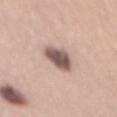notes: no biopsy performed (imaged during a skin exam)
lesion diameter: ≈5 mm
location: the back
imaging modality: 15 mm crop, total-body photography
tile lighting: white-light
automated lesion analysis: a mean CIELAB color near L≈56 a*≈17 b*≈22 and about 17 CIELAB-L* units darker than the surrounding skin
patient: male, approximately 45 years of age